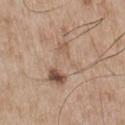<lesion>
<biopsy_status>not biopsied; imaged during a skin examination</biopsy_status>
<lighting>white-light</lighting>
<lesion_size>
  <long_diameter_mm_approx>8.0</long_diameter_mm_approx>
</lesion_size>
<patient>
  <sex>male</sex>
  <age_approx>65</age_approx>
</patient>
<image>
  <source>total-body photography crop</source>
  <field_of_view_mm>15</field_of_view_mm>
</image>
<automated_metrics>
  <area_mm2_approx>16.0</area_mm2_approx>
  <eccentricity>0.9</eccentricity>
  <shape_asymmetry>0.5</shape_asymmetry>
  <cielab_L>57</cielab_L>
  <cielab_a>16</cielab_a>
  <cielab_b>28</cielab_b>
  <vs_skin_darker_L>7.0</vs_skin_darker_L>
  <vs_skin_contrast_norm>5.0</vs_skin_contrast_norm>
  <border_irregularity_0_10>7.0</border_irregularity_0_10>
  <color_variation_0_10>9.0</color_variation_0_10>
  <peripheral_color_asymmetry>3.0</peripheral_color_asymmetry>
</automated_metrics>
<site>chest</site>
</lesion>